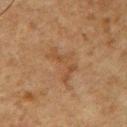Cropped from a total-body skin-imaging series; the visible field is about 15 mm. Automated tile analysis of the lesion measured a footprint of about 8 mm² and a symmetry-axis asymmetry near 0.65. It also reported a lesion color around L≈40 a*≈17 b*≈30 in CIELAB, a lesion–skin lightness drop of about 5, and a lesion-to-skin contrast of about 5 (normalized; higher = more distinct). The analysis additionally found border irregularity of about 8 on a 0–10 scale, internal color variation of about 2 on a 0–10 scale, and peripheral color asymmetry of about 0.5. A male subject, about 60 years old. Measured at roughly 5 mm in maximum diameter. The lesion is on the chest.